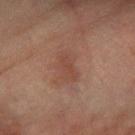Q: Was a biopsy performed?
A: catalogued during a skin exam; not biopsied
Q: How was this image acquired?
A: total-body-photography crop, ~15 mm field of view
Q: Who is the patient?
A: female, about 55 years old
Q: Where on the body is the lesion?
A: the right forearm